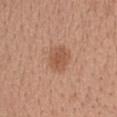biopsy status = imaged on a skin check; not biopsied
patient = female, in their 30s
image source = ~15 mm crop, total-body skin-cancer survey
site = the upper back
illumination = white-light illumination